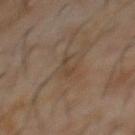Case summary:
– workup: total-body-photography surveillance lesion; no biopsy
– diameter: ≈3 mm
– acquisition: total-body-photography crop, ~15 mm field of view
– subject: male, aged 58 to 62
– location: the front of the torso